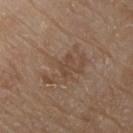Part of a total-body skin-imaging series; this lesion was reviewed on a skin check and was not flagged for biopsy. The subject is a male aged approximately 80. Imaged with white-light lighting. A close-up tile cropped from a whole-body skin photograph, about 15 mm across. The lesion is located on the chest.